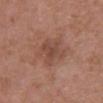Background:
Located on the chest. A roughly 15 mm field-of-view crop from a total-body skin photograph. A female patient aged around 50.